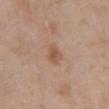Q: Was this lesion biopsied?
A: catalogued during a skin exam; not biopsied
Q: Where on the body is the lesion?
A: the abdomen
Q: Who is the patient?
A: male, aged 78–82
Q: What kind of image is this?
A: total-body-photography crop, ~15 mm field of view